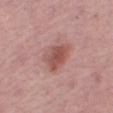notes — catalogued during a skin exam; not biopsied | TBP lesion metrics — a lesion area of about 9 mm² and an outline eccentricity of about 0.7 (0 = round, 1 = elongated); a border-irregularity index near 2.5/10 | subject — female, aged 48 to 52 | lighting — white-light | acquisition — 15 mm crop, total-body photography | body site — the right thigh.